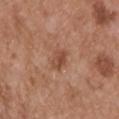Recorded during total-body skin imaging; not selected for excision or biopsy.